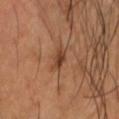<record>
<lighting>cross-polarized</lighting>
<lesion_size>
  <long_diameter_mm_approx>2.5</long_diameter_mm_approx>
</lesion_size>
<patient>
  <sex>male</sex>
  <age_approx>60</age_approx>
</patient>
<site>head or neck</site>
<image>
  <source>total-body photography crop</source>
  <field_of_view_mm>15</field_of_view_mm>
</image>
</record>